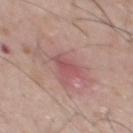  biopsy_status: not biopsied; imaged during a skin examination
  site: upper back
  patient:
    sex: male
    age_approx: 55
  image:
    source: total-body photography crop
    field_of_view_mm: 15
  lesion_size:
    long_diameter_mm_approx: 3.0
  automated_metrics:
    vs_skin_darker_L: 8.0
    color_variation_0_10: 1.5
    peripheral_color_asymmetry: 0.5